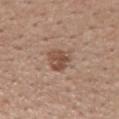notes: imaged on a skin check; not biopsied | image: ~15 mm crop, total-body skin-cancer survey | patient: male, approximately 55 years of age | automated metrics: a footprint of about 7 mm², an eccentricity of roughly 0.55, and two-axis asymmetry of about 0.25; an automated nevus-likeness rating near 50 out of 100 and lesion-presence confidence of about 100/100 | tile lighting: white-light | site: the mid back.